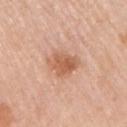  biopsy_status: not biopsied; imaged during a skin examination
  lesion_size:
    long_diameter_mm_approx: 4.5
  site: right upper arm
  lighting: white-light
  patient:
    sex: female
    age_approx: 65
  image:
    source: total-body photography crop
    field_of_view_mm: 15